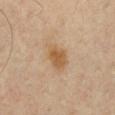biopsy_status: not biopsied; imaged during a skin examination
site: chest
image:
  source: total-body photography crop
  field_of_view_mm: 15
lesion_size:
  long_diameter_mm_approx: 4.0
lighting: cross-polarized
patient:
  sex: male
  age_approx: 40